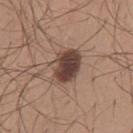notes: imaged on a skin check; not biopsied | lesion diameter: ~4.5 mm (longest diameter) | illumination: white-light | imaging modality: total-body-photography crop, ~15 mm field of view | patient: male, roughly 25 years of age | location: the back.